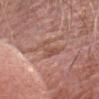Case summary:
* biopsy status — no biopsy performed (imaged during a skin exam)
* body site — the head or neck
* image — ~15 mm crop, total-body skin-cancer survey
* patient — male, aged approximately 65